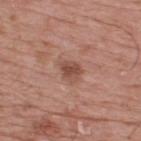workup = no biopsy performed (imaged during a skin exam)
patient = male, aged approximately 75
lesion diameter = about 2.5 mm
acquisition = ~15 mm crop, total-body skin-cancer survey
anatomic site = the upper back